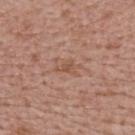follow-up: total-body-photography surveillance lesion; no biopsy
diameter: ~2.5 mm (longest diameter)
subject: female, approximately 65 years of age
image: total-body-photography crop, ~15 mm field of view
site: the upper back
image-analysis metrics: a footprint of about 3 mm² and an eccentricity of roughly 0.75; an automated nevus-likeness rating near 0 out of 100